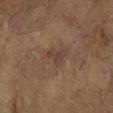The lesion was tiled from a total-body skin photograph and was not biopsied.
A female patient, approximately 80 years of age.
The lesion is located on the left arm.
A 15 mm close-up tile from a total-body photography series done for melanoma screening.